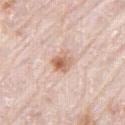Background: The lesion-visualizer software estimated an area of roughly 5.5 mm², an outline eccentricity of about 0.55 (0 = round, 1 = elongated), and a shape-asymmetry score of about 0.2 (0 = symmetric). It also reported a lesion color around L≈65 a*≈19 b*≈30 in CIELAB, roughly 12 lightness units darker than nearby skin, and a lesion-to-skin contrast of about 8 (normalized; higher = more distinct). A male subject aged 78–82. A 15 mm crop from a total-body photograph taken for skin-cancer surveillance. The tile uses white-light illumination. Located on the right thigh. The lesion's longest dimension is about 3 mm.